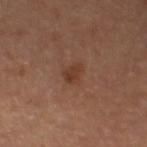Recorded during total-body skin imaging; not selected for excision or biopsy. The lesion-visualizer software estimated a footprint of about 4 mm², a shape eccentricity near 0.8, and two-axis asymmetry of about 0.25. The lesion's longest dimension is about 2.5 mm. Imaged with cross-polarized lighting. From the left thigh. A 15 mm crop from a total-body photograph taken for skin-cancer surveillance. The subject is a male aged approximately 65.